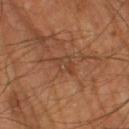follow-up: no biopsy performed (imaged during a skin exam); acquisition: ~15 mm crop, total-body skin-cancer survey; lighting: cross-polarized; diameter: ~3.5 mm (longest diameter); subject: male, approximately 65 years of age; anatomic site: the left lower leg.